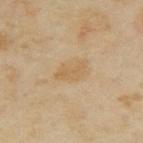Context: Longest diameter approximately 4 mm. A roughly 15 mm field-of-view crop from a total-body skin photograph. The total-body-photography lesion software estimated an area of roughly 5.5 mm² and a shape eccentricity near 0.85. It also reported a lesion-to-skin contrast of about 5.5 (normalized; higher = more distinct). The software also gave a within-lesion color-variation index near 2/10 and a peripheral color-asymmetry measure near 0.5. The lesion is on the upper back. The subject is a female aged around 35. This is a cross-polarized tile.